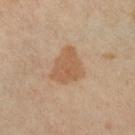Captured during whole-body skin photography for melanoma surveillance; the lesion was not biopsied.
A female subject approximately 50 years of age.
The recorded lesion diameter is about 4.5 mm.
Captured under cross-polarized illumination.
On the right lower leg.
A 15 mm close-up extracted from a 3D total-body photography capture.